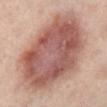{
  "biopsy_status": "not biopsied; imaged during a skin examination",
  "automated_metrics": {
    "border_irregularity_0_10": 2.0,
    "color_variation_0_10": 6.5,
    "peripheral_color_asymmetry": 2.0,
    "nevus_likeness_0_100": 95,
    "lesion_detection_confidence_0_100": 100
  },
  "image": {
    "source": "total-body photography crop",
    "field_of_view_mm": 15
  },
  "site": "left leg",
  "lesion_size": {
    "long_diameter_mm_approx": 10.0
  },
  "lighting": "cross-polarized",
  "patient": {
    "sex": "female",
    "age_approx": 40
  }
}